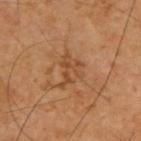| field | value |
|---|---|
| notes | imaged on a skin check; not biopsied |
| image | ~15 mm tile from a whole-body skin photo |
| patient | male, aged approximately 60 |
| location | the upper back |